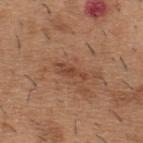  biopsy_status: not biopsied; imaged during a skin examination
  automated_metrics:
    area_mm2_approx: 4.0
    eccentricity: 0.95
  site: upper back
  patient:
    sex: male
    age_approx: 40
  lighting: white-light
  image:
    source: total-body photography crop
    field_of_view_mm: 15
  lesion_size:
    long_diameter_mm_approx: 3.5